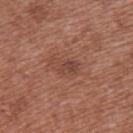From the upper back.
A roughly 15 mm field-of-view crop from a total-body skin photograph.
Imaged with white-light lighting.
The subject is a female in their 40s.